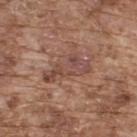Clinical impression:
The lesion was photographed on a routine skin check and not biopsied; there is no pathology result.